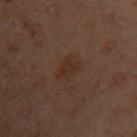{
  "biopsy_status": "not biopsied; imaged during a skin examination"
}